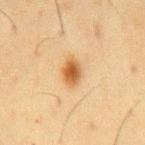biopsy status = catalogued during a skin exam; not biopsied | image source = 15 mm crop, total-body photography | patient = male, aged 58 to 62 | automated metrics = a footprint of about 6 mm², a shape eccentricity near 0.7, and a symmetry-axis asymmetry near 0.2; border irregularity of about 2 on a 0–10 scale, a color-variation rating of about 4.5/10, and radial color variation of about 1.5; a nevus-likeness score of about 100/100 and a detector confidence of about 100 out of 100 that the crop contains a lesion | size = ≈3.5 mm | anatomic site = the chest | tile lighting = cross-polarized illumination.